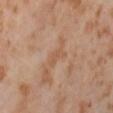| field | value |
|---|---|
| image source | ~15 mm tile from a whole-body skin photo |
| lesion diameter | ~3 mm (longest diameter) |
| image-analysis metrics | an eccentricity of roughly 0.85 and a shape-asymmetry score of about 0.35 (0 = symmetric); a border-irregularity rating of about 3.5/10, a color-variation rating of about 0.5/10, and radial color variation of about 0 |
| location | the abdomen |
| lighting | cross-polarized illumination |
| patient | female, aged approximately 55 |